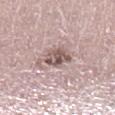Recorded during total-body skin imaging; not selected for excision or biopsy.